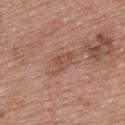biopsy status: total-body-photography surveillance lesion; no biopsy